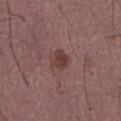biopsy_status: not biopsied; imaged during a skin examination